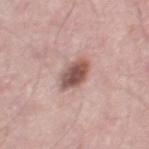lesion size = ≈3.5 mm
site = the right thigh
acquisition = ~15 mm crop, total-body skin-cancer survey
patient = male, roughly 60 years of age
TBP lesion metrics = border irregularity of about 1.5 on a 0–10 scale, internal color variation of about 5 on a 0–10 scale, and radial color variation of about 1.5; a classifier nevus-likeness of about 80/100 and a lesion-detection confidence of about 100/100
lighting = white-light